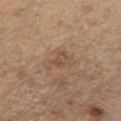Q: Was a biopsy performed?
A: no biopsy performed (imaged during a skin exam)
Q: What are the patient's age and sex?
A: male, aged approximately 70
Q: How was the tile lit?
A: white-light illumination
Q: What is the anatomic site?
A: the chest
Q: How large is the lesion?
A: about 3.5 mm
Q: What is the imaging modality?
A: ~15 mm crop, total-body skin-cancer survey